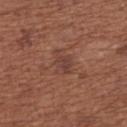Imaged during a routine full-body skin examination; the lesion was not biopsied and no histopathology is available.
Located on the back.
A lesion tile, about 15 mm wide, cut from a 3D total-body photograph.
Imaged with white-light lighting.
The patient is a female approximately 65 years of age.
Measured at roughly 3.5 mm in maximum diameter.